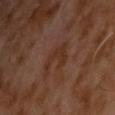| feature | finding |
|---|---|
| follow-up | no biopsy performed (imaged during a skin exam) |
| image source | total-body-photography crop, ~15 mm field of view |
| lighting | cross-polarized |
| patient | male, roughly 60 years of age |
| site | the chest |